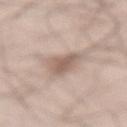Clinical impression: No biopsy was performed on this lesion — it was imaged during a full skin examination and was not determined to be concerning. Context: The total-body-photography lesion software estimated a lesion area of about 7.5 mm², an eccentricity of roughly 0.85, and two-axis asymmetry of about 0.3. The software also gave an automated nevus-likeness rating near 40 out of 100 and a lesion-detection confidence of about 75/100. The lesion is located on the back. This image is a 15 mm lesion crop taken from a total-body photograph. The patient is a male in their mid- to late 30s. The lesion's longest dimension is about 4.5 mm. This is a white-light tile.